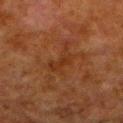biopsy status — catalogued during a skin exam; not biopsied | diameter — ~4.5 mm (longest diameter) | tile lighting — cross-polarized | image — ~15 mm crop, total-body skin-cancer survey | TBP lesion metrics — a lesion area of about 7 mm², an eccentricity of roughly 0.8, and a symmetry-axis asymmetry near 0.5; roughly 5 lightness units darker than nearby skin and a normalized border contrast of about 5.5; a border-irregularity index near 7/10 and internal color variation of about 2 on a 0–10 scale; a classifier nevus-likeness of about 0/100 | anatomic site — the left lower leg | subject — male, roughly 80 years of age.